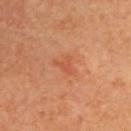Part of a total-body skin-imaging series; this lesion was reviewed on a skin check and was not flagged for biopsy.